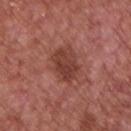Imaged during a routine full-body skin examination; the lesion was not biopsied and no histopathology is available. A roughly 15 mm field-of-view crop from a total-body skin photograph. The lesion is on the chest. A male patient in their mid-60s.